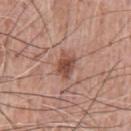Assessment:
No biopsy was performed on this lesion — it was imaged during a full skin examination and was not determined to be concerning.
Image and clinical context:
The total-body-photography lesion software estimated a footprint of about 5.5 mm², a shape eccentricity near 0.75, and a symmetry-axis asymmetry near 0.25. It also reported border irregularity of about 3 on a 0–10 scale. The software also gave a nevus-likeness score of about 85/100 and a lesion-detection confidence of about 100/100. A close-up tile cropped from a whole-body skin photograph, about 15 mm across. A male subject roughly 60 years of age. On the chest.